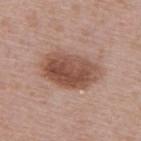{"biopsy_status": "not biopsied; imaged during a skin examination", "image": {"source": "total-body photography crop", "field_of_view_mm": 15}, "site": "upper back", "patient": {"sex": "female", "age_approx": 60}, "lesion_size": {"long_diameter_mm_approx": 6.5}, "lighting": "white-light"}